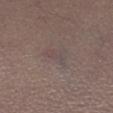image:
  source: total-body photography crop
  field_of_view_mm: 15
lesion_size:
  long_diameter_mm_approx: 3.5
patient:
  sex: male
  age_approx: 40
site: left lower leg
automated_metrics:
  border_irregularity_0_10: 4.0
  color_variation_0_10: 2.5
  peripheral_color_asymmetry: 1.0
lighting: white-light